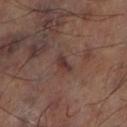Context: Located on the left thigh. Captured under cross-polarized illumination. Longest diameter approximately 2.5 mm. A 15 mm close-up extracted from a 3D total-body photography capture.